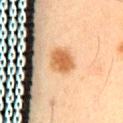Imaged during a routine full-body skin examination; the lesion was not biopsied and no histopathology is available. The lesion's longest dimension is about 3.5 mm. Captured under cross-polarized illumination. A male subject, aged 63 to 67. The lesion is on the lower back. A 15 mm close-up tile from a total-body photography series done for melanoma screening.